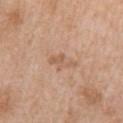Captured during whole-body skin photography for melanoma surveillance; the lesion was not biopsied. Automated tile analysis of the lesion measured an outline eccentricity of about 0.9 (0 = round, 1 = elongated) and a shape-asymmetry score of about 0.4 (0 = symmetric). The lesion is on the right upper arm. A female patient, aged around 55. Captured under white-light illumination. A 15 mm close-up extracted from a 3D total-body photography capture.